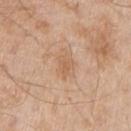This lesion was catalogued during total-body skin photography and was not selected for biopsy. This is a white-light tile. A male subject, aged 63–67. The lesion is on the left upper arm. Longest diameter approximately 2.5 mm. A 15 mm crop from a total-body photograph taken for skin-cancer surveillance.